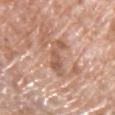biopsy_status: not biopsied; imaged during a skin examination
lesion_size:
  long_diameter_mm_approx: 5.0
automated_metrics:
  area_mm2_approx: 6.5
  eccentricity: 0.95
  shape_asymmetry: 0.45
  cielab_L: 57
  cielab_a: 21
  cielab_b: 30
  border_irregularity_0_10: 7.0
  color_variation_0_10: 2.0
  peripheral_color_asymmetry: 0.5
  nevus_likeness_0_100: 0
image:
  source: total-body photography crop
  field_of_view_mm: 15
patient:
  sex: male
  age_approx: 70
lighting: white-light
site: left upper arm